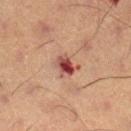Notes:
– follow-up — imaged on a skin check; not biopsied
– image — total-body-photography crop, ~15 mm field of view
– automated metrics — an area of roughly 4.5 mm², an eccentricity of roughly 0.6, and two-axis asymmetry of about 0.25; a lesion color around L≈41 a*≈26 b*≈23 in CIELAB, a lesion–skin lightness drop of about 14, and a lesion-to-skin contrast of about 11 (normalized; higher = more distinct); a nevus-likeness score of about 0/100 and a detector confidence of about 100 out of 100 that the crop contains a lesion
– lighting — cross-polarized
– subject — male, about 55 years old
– anatomic site — the left lower leg
– lesion diameter — ~2.5 mm (longest diameter)